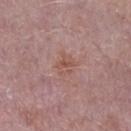workup=catalogued during a skin exam; not biopsied | tile lighting=white-light | location=the left lower leg | lesion size=~3 mm (longest diameter) | imaging modality=15 mm crop, total-body photography | patient=male, aged 73 to 77.